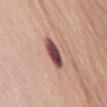Q: Was this lesion biopsied?
A: imaged on a skin check; not biopsied
Q: Lesion location?
A: the chest
Q: Patient demographics?
A: female, about 70 years old
Q: How was this image acquired?
A: total-body-photography crop, ~15 mm field of view